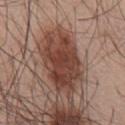Findings:
* workup — no biopsy performed (imaged during a skin exam)
* subject — male, aged approximately 55
* diameter — ~7 mm (longest diameter)
* TBP lesion metrics — an automated nevus-likeness rating near 90 out of 100 and a detector confidence of about 100 out of 100 that the crop contains a lesion
* anatomic site — the back
* imaging modality — ~15 mm tile from a whole-body skin photo
* tile lighting — white-light